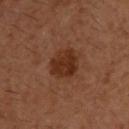Recorded during total-body skin imaging; not selected for excision or biopsy.
A male patient, about 30 years old.
Longest diameter approximately 3.5 mm.
The lesion is on the upper back.
A roughly 15 mm field-of-view crop from a total-body skin photograph.